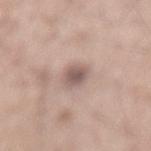Clinical impression:
Captured during whole-body skin photography for melanoma surveillance; the lesion was not biopsied.
Context:
The lesion-visualizer software estimated a footprint of about 5 mm². It also reported a color-variation rating of about 3/10 and radial color variation of about 0.5. It also reported an automated nevus-likeness rating near 10 out of 100 and lesion-presence confidence of about 100/100. The subject is a male about 60 years old. About 3 mm across. On the lower back. A 15 mm crop from a total-body photograph taken for skin-cancer surveillance. Imaged with white-light lighting.